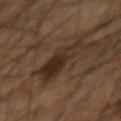automated metrics: an area of roughly 14 mm², an outline eccentricity of about 0.8 (0 = round, 1 = elongated), and a symmetry-axis asymmetry near 0.45; a mean CIELAB color near L≈22 a*≈12 b*≈19 and about 7 CIELAB-L* units darker than the surrounding skin; a within-lesion color-variation index near 4.5/10 and a peripheral color-asymmetry measure near 1.5; a detector confidence of about 85 out of 100 that the crop contains a lesion | body site: the chest | tile lighting: cross-polarized illumination | imaging modality: ~15 mm tile from a whole-body skin photo | subject: male, in their mid- to late 60s.